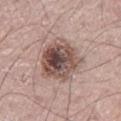notes: total-body-photography surveillance lesion; no biopsy | lighting: white-light illumination | lesion size: ~5.5 mm (longest diameter) | imaging modality: ~15 mm tile from a whole-body skin photo | body site: the right leg | subject: male, roughly 70 years of age.